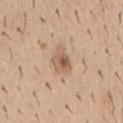Captured during whole-body skin photography for melanoma surveillance; the lesion was not biopsied. Located on the abdomen. A close-up tile cropped from a whole-body skin photograph, about 15 mm across. A male subject in their 40s.